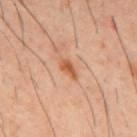workup: imaged on a skin check; not biopsied
imaging modality: ~15 mm crop, total-body skin-cancer survey
subject: male, aged approximately 40
location: the mid back
automated lesion analysis: a footprint of about 3 mm² and an outline eccentricity of about 0.85 (0 = round, 1 = elongated); a lesion–skin lightness drop of about 11 and a normalized lesion–skin contrast near 8; an automated nevus-likeness rating near 55 out of 100 and a lesion-detection confidence of about 100/100
illumination: cross-polarized